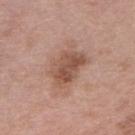Q: Was a biopsy performed?
A: total-body-photography surveillance lesion; no biopsy
Q: What did automated image analysis measure?
A: a lesion area of about 12 mm², an eccentricity of roughly 0.75, and two-axis asymmetry of about 0.3; border irregularity of about 3.5 on a 0–10 scale, a color-variation rating of about 4.5/10, and radial color variation of about 1.5; an automated nevus-likeness rating near 10 out of 100 and lesion-presence confidence of about 100/100
Q: Who is the patient?
A: female, approximately 45 years of age
Q: Lesion size?
A: ≈5 mm
Q: What is the imaging modality?
A: ~15 mm crop, total-body skin-cancer survey
Q: Lesion location?
A: the right upper arm